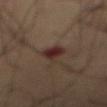notes: no biopsy performed (imaged during a skin exam) | body site: the abdomen | subject: male, aged around 70 | diameter: ~3.5 mm (longest diameter) | acquisition: ~15 mm tile from a whole-body skin photo | illumination: cross-polarized illumination.